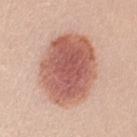notes: catalogued during a skin exam; not biopsied | TBP lesion metrics: an average lesion color of about L≈58 a*≈25 b*≈27 (CIELAB), roughly 16 lightness units darker than nearby skin, and a lesion-to-skin contrast of about 9.5 (normalized; higher = more distinct); a border-irregularity rating of about 1.5/10, internal color variation of about 5.5 on a 0–10 scale, and radial color variation of about 1.5 | tile lighting: white-light | subject: female, aged 23–27 | lesion diameter: ≈8.5 mm | image: total-body-photography crop, ~15 mm field of view | body site: the left upper arm.